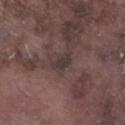Notes:
- biopsy status: imaged on a skin check; not biopsied
- image source: total-body-photography crop, ~15 mm field of view
- location: the left lower leg
- image-analysis metrics: a border-irregularity index near 2/10, internal color variation of about 1 on a 0–10 scale, and peripheral color asymmetry of about 0.5; an automated nevus-likeness rating near 0 out of 100 and a lesion-detection confidence of about 55/100
- lesion diameter: ~2.5 mm (longest diameter)
- patient: male, aged around 75